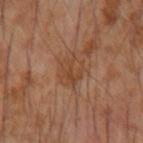{
  "biopsy_status": "not biopsied; imaged during a skin examination",
  "image": {
    "source": "total-body photography crop",
    "field_of_view_mm": 15
  },
  "patient": {
    "sex": "male",
    "age_approx": 55
  },
  "lesion_size": {
    "long_diameter_mm_approx": 3.5
  },
  "site": "right forearm"
}